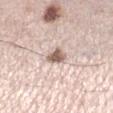follow-up: catalogued during a skin exam; not biopsied
location: the right lower leg
size: ~2.5 mm (longest diameter)
patient: female, aged around 45
image source: ~15 mm crop, total-body skin-cancer survey